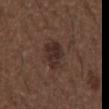biopsy status: imaged on a skin check; not biopsied | subject: male, aged 48–52 | site: the right forearm | acquisition: total-body-photography crop, ~15 mm field of view.